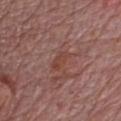Findings:
* notes · no biopsy performed (imaged during a skin exam)
* anatomic site · the front of the torso
* patient · male, in their mid- to late 70s
* lesion size · ≈3 mm
* imaging modality · 15 mm crop, total-body photography
* illumination · white-light